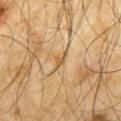Assessment:
Imaged during a routine full-body skin examination; the lesion was not biopsied and no histopathology is available.
Acquisition and patient details:
Approximately 2.5 mm at its widest. The lesion is on the back. Automated image analysis of the tile measured a lesion color around L≈55 a*≈16 b*≈38 in CIELAB, roughly 9 lightness units darker than nearby skin, and a normalized lesion–skin contrast near 6.5. The software also gave a border-irregularity rating of about 5/10, internal color variation of about 0 on a 0–10 scale, and a peripheral color-asymmetry measure near 0. And it measured a classifier nevus-likeness of about 0/100 and a detector confidence of about 65 out of 100 that the crop contains a lesion. Cropped from a total-body skin-imaging series; the visible field is about 15 mm. The tile uses cross-polarized illumination. A male subject, roughly 65 years of age.